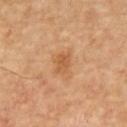notes = imaged on a skin check; not biopsied
subject = male, aged around 65
lesion size = ~2.5 mm (longest diameter)
automated metrics = a footprint of about 4.5 mm² and a shape eccentricity near 0.75; an average lesion color of about L≈55 a*≈23 b*≈38 (CIELAB), a lesion–skin lightness drop of about 8, and a normalized border contrast of about 6; a nevus-likeness score of about 0/100 and a detector confidence of about 100 out of 100 that the crop contains a lesion
image source = total-body-photography crop, ~15 mm field of view